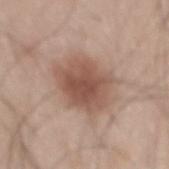Case summary:
- tile lighting · white-light illumination
- subject · male, about 45 years old
- anatomic site · the mid back
- image source · ~15 mm crop, total-body skin-cancer survey
- automated metrics · a footprint of about 22 mm², an outline eccentricity of about 0.65 (0 = round, 1 = elongated), and two-axis asymmetry of about 0.2; a border-irregularity rating of about 2.5/10 and a peripheral color-asymmetry measure near 1